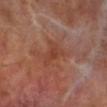The lesion was tiled from a total-body skin photograph and was not biopsied.
Measured at roughly 3.5 mm in maximum diameter.
Located on the leg.
A close-up tile cropped from a whole-body skin photograph, about 15 mm across.
The tile uses cross-polarized illumination.
The subject is a male roughly 70 years of age.
Automated image analysis of the tile measured a border-irregularity index near 5/10, a color-variation rating of about 1.5/10, and peripheral color asymmetry of about 0.5. It also reported a lesion-detection confidence of about 100/100.